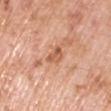Imaged during a routine full-body skin examination; the lesion was not biopsied and no histopathology is available.
The subject is a male aged 58–62.
A close-up tile cropped from a whole-body skin photograph, about 15 mm across.
This is a white-light tile.
Approximately 3 mm at its widest.
Automated image analysis of the tile measured a lesion color around L≈58 a*≈26 b*≈34 in CIELAB, about 10 CIELAB-L* units darker than the surrounding skin, and a normalized lesion–skin contrast near 6.5. The software also gave border irregularity of about 4 on a 0–10 scale.
Located on the right upper arm.